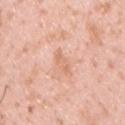Imaged during a routine full-body skin examination; the lesion was not biopsied and no histopathology is available. Cropped from a whole-body photographic skin survey; the tile spans about 15 mm. The subject is a male aged 33–37. Longest diameter approximately 3.5 mm. Located on the upper back. The tile uses white-light illumination.